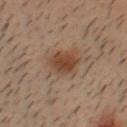Impression:
No biopsy was performed on this lesion — it was imaged during a full skin examination and was not determined to be concerning.
Background:
A lesion tile, about 15 mm wide, cut from a 3D total-body photograph. A male patient, aged around 30. The lesion is on the head or neck. The total-body-photography lesion software estimated a border-irregularity rating of about 2.5/10, a within-lesion color-variation index near 4/10, and peripheral color asymmetry of about 1. The software also gave lesion-presence confidence of about 100/100. The lesion's longest dimension is about 4 mm. Captured under cross-polarized illumination.